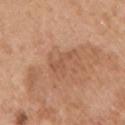- follow-up: imaged on a skin check; not biopsied
- location: the right upper arm
- image: total-body-photography crop, ~15 mm field of view
- patient: male, roughly 60 years of age
- tile lighting: white-light
- size: ~4 mm (longest diameter)
- automated metrics: a mean CIELAB color near L≈54 a*≈22 b*≈33, roughly 6 lightness units darker than nearby skin, and a normalized lesion–skin contrast near 4.5; a border-irregularity index near 7.5/10 and internal color variation of about 0.5 on a 0–10 scale; an automated nevus-likeness rating near 0 out of 100 and a lesion-detection confidence of about 100/100